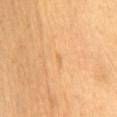Assessment: Imaged during a routine full-body skin examination; the lesion was not biopsied and no histopathology is available. Image and clinical context: The recorded lesion diameter is about 1 mm. The patient is a female about 40 years old. The lesion is on the mid back. The total-body-photography lesion software estimated a lesion area of about 0.5 mm², an eccentricity of roughly 0.85, and a shape-asymmetry score of about 0.45 (0 = symmetric). It also reported an average lesion color of about L≈67 a*≈23 b*≈45 (CIELAB), about 6 CIELAB-L* units darker than the surrounding skin, and a normalized border contrast of about 4. The tile uses cross-polarized illumination. A region of skin cropped from a whole-body photographic capture, roughly 15 mm wide.